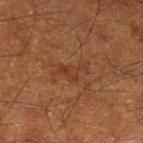Assessment: The lesion was photographed on a routine skin check and not biopsied; there is no pathology result. Image and clinical context: Located on the leg. Automated tile analysis of the lesion measured a lesion color around L≈30 a*≈19 b*≈27 in CIELAB and a normalized border contrast of about 5. The software also gave a border-irregularity index near 8.5/10, a within-lesion color-variation index near 2/10, and peripheral color asymmetry of about 0.5. A roughly 15 mm field-of-view crop from a total-body skin photograph. The patient is a male in their mid- to late 60s. Imaged with cross-polarized lighting. About 4 mm across.